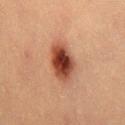This is a cross-polarized tile. Cropped from a total-body skin-imaging series; the visible field is about 15 mm. The recorded lesion diameter is about 5 mm. A male patient, in their 40s. From the right thigh.